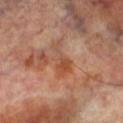biopsy status=total-body-photography surveillance lesion; no biopsy
site=the left lower leg
acquisition=~15 mm crop, total-body skin-cancer survey
subject=male, aged around 70